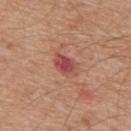Q: Was this lesion biopsied?
A: imaged on a skin check; not biopsied